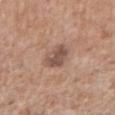Q: Was a biopsy performed?
A: no biopsy performed (imaged during a skin exam)
Q: What is the anatomic site?
A: the right upper arm
Q: Patient demographics?
A: male, roughly 65 years of age
Q: How was the tile lit?
A: white-light illumination
Q: What kind of image is this?
A: 15 mm crop, total-body photography
Q: How large is the lesion?
A: ≈4 mm